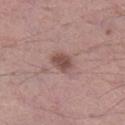{
  "biopsy_status": "not biopsied; imaged during a skin examination",
  "patient": {
    "sex": "male",
    "age_approx": 55
  },
  "site": "right lower leg",
  "image": {
    "source": "total-body photography crop",
    "field_of_view_mm": 15
  },
  "lighting": "white-light"
}